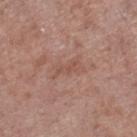Notes:
- follow-up: imaged on a skin check; not biopsied
- image source: total-body-photography crop, ~15 mm field of view
- tile lighting: white-light illumination
- site: the right lower leg
- subject: male, in their mid- to late 50s
- diameter: ~4 mm (longest diameter)